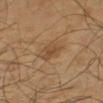Clinical impression: Part of a total-body skin-imaging series; this lesion was reviewed on a skin check and was not flagged for biopsy. Clinical summary: The subject is a male in their 60s. The tile uses cross-polarized illumination. Located on the right arm. Automated image analysis of the tile measured about 7 CIELAB-L* units darker than the surrounding skin and a normalized lesion–skin contrast near 6. The analysis additionally found a border-irregularity rating of about 3.5/10 and peripheral color asymmetry of about 1. Approximately 3 mm at its widest. A 15 mm crop from a total-body photograph taken for skin-cancer surveillance.